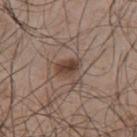biopsy_status: not biopsied; imaged during a skin examination
image:
  source: total-body photography crop
  field_of_view_mm: 15
patient:
  sex: male
  age_approx: 50
site: upper back